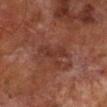notes: imaged on a skin check; not biopsied
size: ≈4.5 mm
location: the right lower leg
imaging modality: ~15 mm crop, total-body skin-cancer survey
patient: male, aged 68 to 72
lighting: cross-polarized illumination